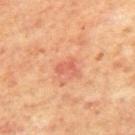Assessment: Captured during whole-body skin photography for melanoma surveillance; the lesion was not biopsied. Context: The tile uses cross-polarized illumination. A 15 mm crop from a total-body photograph taken for skin-cancer surveillance. The lesion is on the back. The lesion's longest dimension is about 2.5 mm. A male patient approximately 65 years of age.